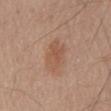Image and clinical context: Imaged with white-light lighting. About 5 mm across. A male patient, about 65 years old. The lesion-visualizer software estimated a lesion-detection confidence of about 100/100. Cropped from a total-body skin-imaging series; the visible field is about 15 mm. The lesion is located on the mid back.